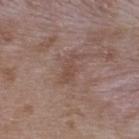• follow-up · total-body-photography surveillance lesion; no biopsy
• subject · male, approximately 50 years of age
• site · the upper back
• acquisition · total-body-photography crop, ~15 mm field of view
• lesion diameter · about 3.5 mm